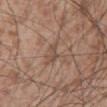About 2.5 mm across.
A 15 mm close-up extracted from a 3D total-body photography capture.
Automated tile analysis of the lesion measured a shape eccentricity near 0.9. And it measured a mean CIELAB color near L≈49 a*≈17 b*≈26, about 7 CIELAB-L* units darker than the surrounding skin, and a normalized lesion–skin contrast near 5.5. The analysis additionally found border irregularity of about 3 on a 0–10 scale, a within-lesion color-variation index near 1/10, and a peripheral color-asymmetry measure near 0.5. It also reported a classifier nevus-likeness of about 0/100 and lesion-presence confidence of about 60/100.
Located on the abdomen.
The subject is a male approximately 55 years of age.